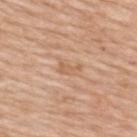| field | value |
|---|---|
| TBP lesion metrics | a footprint of about 2.5 mm², an outline eccentricity of about 0.9 (0 = round, 1 = elongated), and two-axis asymmetry of about 0.6; about 7 CIELAB-L* units darker than the surrounding skin and a normalized lesion–skin contrast near 5.5; a classifier nevus-likeness of about 0/100 and a lesion-detection confidence of about 100/100 |
| patient | female, about 45 years old |
| illumination | white-light illumination |
| image source | 15 mm crop, total-body photography |
| site | the upper back |